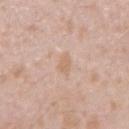Part of a total-body skin-imaging series; this lesion was reviewed on a skin check and was not flagged for biopsy. This image is a 15 mm lesion crop taken from a total-body photograph. From the right upper arm. A male patient, aged 23–27.